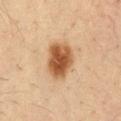Case summary:
– notes · catalogued during a skin exam; not biopsied
– lesion size · ~4.5 mm (longest diameter)
– automated metrics · a lesion area of about 12 mm², an eccentricity of roughly 0.65, and a shape-asymmetry score of about 0.2 (0 = symmetric); a mean CIELAB color near L≈50 a*≈21 b*≈35 and about 16 CIELAB-L* units darker than the surrounding skin; a classifier nevus-likeness of about 100/100 and lesion-presence confidence of about 100/100
– subject · male, in their mid- to late 30s
– location · the front of the torso
– image · 15 mm crop, total-body photography
– illumination · cross-polarized illumination